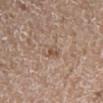<case>
  <biopsy_status>not biopsied; imaged during a skin examination</biopsy_status>
  <patient>
    <sex>female</sex>
    <age_approx>70</age_approx>
  </patient>
  <image>
    <source>total-body photography crop</source>
    <field_of_view_mm>15</field_of_view_mm>
  </image>
  <site>right lower leg</site>
  <lesion_size>
    <long_diameter_mm_approx>3.5</long_diameter_mm_approx>
  </lesion_size>
  <lighting>white-light</lighting>
</case>